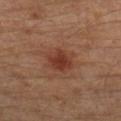Notes:
– notes — no biopsy performed (imaged during a skin exam)
– body site — the left leg
– lighting — cross-polarized
– acquisition — total-body-photography crop, ~15 mm field of view
– lesion diameter — about 4 mm
– image-analysis metrics — a color-variation rating of about 2.5/10 and peripheral color asymmetry of about 0.5; an automated nevus-likeness rating near 90 out of 100
– subject — male, aged around 30